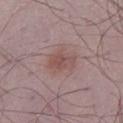Clinical impression:
Part of a total-body skin-imaging series; this lesion was reviewed on a skin check and was not flagged for biopsy.
Acquisition and patient details:
Cropped from a whole-body photographic skin survey; the tile spans about 15 mm. A male subject aged around 50. Longest diameter approximately 3.5 mm. Automated tile analysis of the lesion measured a lesion color around L≈50 a*≈19 b*≈21 in CIELAB, about 7 CIELAB-L* units darker than the surrounding skin, and a normalized lesion–skin contrast near 6. And it measured border irregularity of about 2.5 on a 0–10 scale, a color-variation rating of about 3.5/10, and a peripheral color-asymmetry measure near 1. It also reported lesion-presence confidence of about 100/100. On the left lower leg. This is a white-light tile.